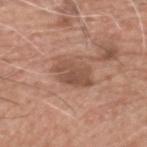The lesion was tiled from a total-body skin photograph and was not biopsied.
The recorded lesion diameter is about 4.5 mm.
The subject is a male aged approximately 75.
On the head or neck.
This is a white-light tile.
A 15 mm close-up extracted from a 3D total-body photography capture.